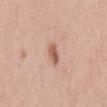biopsy status = no biopsy performed (imaged during a skin exam) | acquisition = total-body-photography crop, ~15 mm field of view | site = the lower back | patient = female, aged 43 to 47 | automated lesion analysis = an average lesion color of about L≈59 a*≈22 b*≈31 (CIELAB), a lesion–skin lightness drop of about 12, and a normalized lesion–skin contrast near 8; a nevus-likeness score of about 90/100 and a lesion-detection confidence of about 100/100 | illumination = white-light illumination.